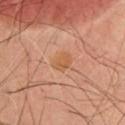The lesion was tiled from a total-body skin photograph and was not biopsied.
The recorded lesion diameter is about 2.5 mm.
A male patient about 55 years old.
A 15 mm close-up tile from a total-body photography series done for melanoma screening.
The tile uses cross-polarized illumination.
On the chest.